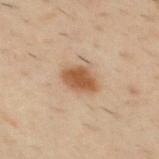Impression: Imaged during a routine full-body skin examination; the lesion was not biopsied and no histopathology is available. Acquisition and patient details: Captured under cross-polarized illumination. A close-up tile cropped from a whole-body skin photograph, about 15 mm across. The lesion-visualizer software estimated a mean CIELAB color near L≈54 a*≈20 b*≈35. The analysis additionally found a nevus-likeness score of about 100/100 and a detector confidence of about 100 out of 100 that the crop contains a lesion. Approximately 3.5 mm at its widest. On the back. The subject is a male aged 38 to 42.